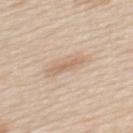Imaged during a routine full-body skin examination; the lesion was not biopsied and no histopathology is available.
A 15 mm close-up tile from a total-body photography series done for melanoma screening.
On the back.
The patient is a male aged around 60.